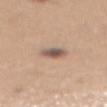Notes:
- biopsy status — catalogued during a skin exam; not biopsied
- illumination — white-light illumination
- body site — the mid back
- image source — ~15 mm tile from a whole-body skin photo
- diameter — ≈3 mm
- subject — female, about 30 years old